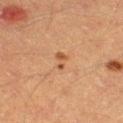notes: catalogued during a skin exam; not biopsied | patient: male, aged around 40 | lesion size: ≈3 mm | tile lighting: cross-polarized | acquisition: ~15 mm crop, total-body skin-cancer survey | anatomic site: the leg.